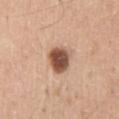The lesion was tiled from a total-body skin photograph and was not biopsied.